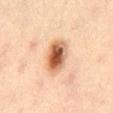follow-up = catalogued during a skin exam; not biopsied | size = about 4.5 mm | lighting = cross-polarized | location = the abdomen | image = ~15 mm tile from a whole-body skin photo | patient = male, roughly 45 years of age.